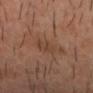Q: How large is the lesion?
A: ~2.5 mm (longest diameter)
Q: How was this image acquired?
A: 15 mm crop, total-body photography
Q: Patient demographics?
A: male, aged 38 to 42
Q: What did automated image analysis measure?
A: a lesion color around L≈40 a*≈18 b*≈28 in CIELAB, a lesion–skin lightness drop of about 5, and a normalized lesion–skin contrast near 4.5; a nevus-likeness score of about 0/100
Q: What is the anatomic site?
A: the head or neck
Q: What lighting was used for the tile?
A: cross-polarized illumination